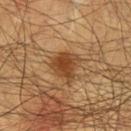workup: total-body-photography surveillance lesion; no biopsy
lesion size: about 3.5 mm
image: ~15 mm tile from a whole-body skin photo
illumination: cross-polarized illumination
location: the chest
patient: male, aged approximately 60